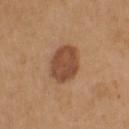Assessment:
Part of a total-body skin-imaging series; this lesion was reviewed on a skin check and was not flagged for biopsy.
Acquisition and patient details:
A female subject aged 38 to 42. A roughly 15 mm field-of-view crop from a total-body skin photograph. This is a white-light tile. Automated image analysis of the tile measured about 12 CIELAB-L* units darker than the surrounding skin and a lesion-to-skin contrast of about 8.5 (normalized; higher = more distinct). And it measured a nevus-likeness score of about 85/100 and a lesion-detection confidence of about 100/100. From the left upper arm.